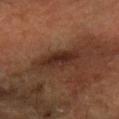Clinical impression:
The lesion was photographed on a routine skin check and not biopsied; there is no pathology result.
Acquisition and patient details:
This image is a 15 mm lesion crop taken from a total-body photograph. Measured at roughly 5 mm in maximum diameter. This is a cross-polarized tile. A male patient aged 58–62. The lesion is located on the right forearm.